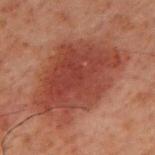Notes:
* notes · catalogued during a skin exam; not biopsied